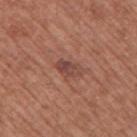Impression: Imaged during a routine full-body skin examination; the lesion was not biopsied and no histopathology is available. Context: Located on the right upper arm. Imaged with white-light lighting. Cropped from a total-body skin-imaging series; the visible field is about 15 mm. A male subject approximately 75 years of age. Automated tile analysis of the lesion measured a mean CIELAB color near L≈45 a*≈23 b*≈25 and a lesion–skin lightness drop of about 9. The analysis additionally found internal color variation of about 4 on a 0–10 scale and peripheral color asymmetry of about 1.5. It also reported a nevus-likeness score of about 15/100 and a detector confidence of about 100 out of 100 that the crop contains a lesion. About 3 mm across.